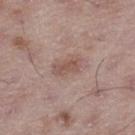<tbp_lesion>
<biopsy_status>not biopsied; imaged during a skin examination</biopsy_status>
<image>
  <source>total-body photography crop</source>
  <field_of_view_mm>15</field_of_view_mm>
</image>
<site>left thigh</site>
<patient>
  <sex>male</sex>
  <age_approx>50</age_approx>
</patient>
<lesion_size>
  <long_diameter_mm_approx>3.5</long_diameter_mm_approx>
</lesion_size>
<lighting>white-light</lighting>
</tbp_lesion>